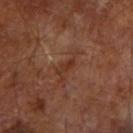biopsy status=imaged on a skin check; not biopsied